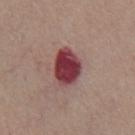Recorded during total-body skin imaging; not selected for excision or biopsy. Cropped from a whole-body photographic skin survey; the tile spans about 15 mm. Longest diameter approximately 4.5 mm. Automated tile analysis of the lesion measured a footprint of about 12 mm², a shape eccentricity near 0.7, and two-axis asymmetry of about 0.2. The software also gave a mean CIELAB color near L≈40 a*≈29 b*≈19 and roughly 18 lightness units darker than nearby skin. The analysis additionally found internal color variation of about 5.5 on a 0–10 scale. The software also gave a classifier nevus-likeness of about 0/100. The lesion is on the front of the torso. The subject is a male about 55 years old. Imaged with white-light lighting.